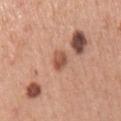Assessment: No biopsy was performed on this lesion — it was imaged during a full skin examination and was not determined to be concerning. Acquisition and patient details: A roughly 15 mm field-of-view crop from a total-body skin photograph. Located on the right upper arm. The subject is a male in their mid- to late 50s.